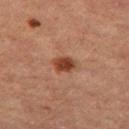Imaged during a routine full-body skin examination; the lesion was not biopsied and no histopathology is available.
A 15 mm close-up extracted from a 3D total-body photography capture.
Captured under cross-polarized illumination.
The lesion is located on the left thigh.
A female subject, in their 60s.